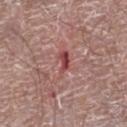biopsy status: imaged on a skin check; not biopsied
lighting: white-light
imaging modality: 15 mm crop, total-body photography
automated metrics: a footprint of about 6 mm², a shape eccentricity near 0.85, and a shape-asymmetry score of about 0.65 (0 = symmetric); border irregularity of about 8 on a 0–10 scale and peripheral color asymmetry of about 2; a lesion-detection confidence of about 100/100
patient: male, about 65 years old
site: the leg